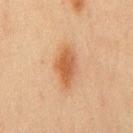  biopsy_status: not biopsied; imaged during a skin examination
  image:
    source: total-body photography crop
    field_of_view_mm: 15
  patient:
    sex: male
    age_approx: 45
  lighting: cross-polarized
  lesion_size:
    long_diameter_mm_approx: 5.0
  site: chest